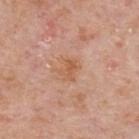Part of a total-body skin-imaging series; this lesion was reviewed on a skin check and was not flagged for biopsy. The subject is a male aged approximately 75. Approximately 3 mm at its widest. The lesion is on the upper back. The lesion-visualizer software estimated an eccentricity of roughly 0.6 and a shape-asymmetry score of about 0.2 (0 = symmetric). The analysis additionally found a mean CIELAB color near L≈58 a*≈23 b*≈35, a lesion–skin lightness drop of about 7, and a lesion-to-skin contrast of about 6 (normalized; higher = more distinct). And it measured a border-irregularity rating of about 2.5/10 and radial color variation of about 1. Imaged with white-light lighting. A lesion tile, about 15 mm wide, cut from a 3D total-body photograph.